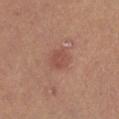The lesion is located on the right lower leg.
Longest diameter approximately 2.5 mm.
The subject is a female in their mid- to late 40s.
This is a cross-polarized tile.
A 15 mm close-up tile from a total-body photography series done for melanoma screening.